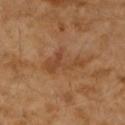<case>
<biopsy_status>not biopsied; imaged during a skin examination</biopsy_status>
<site>right forearm</site>
<lesion_size>
  <long_diameter_mm_approx>5.5</long_diameter_mm_approx>
</lesion_size>
<image>
  <source>total-body photography crop</source>
  <field_of_view_mm>15</field_of_view_mm>
</image>
<lighting>cross-polarized</lighting>
<patient>
  <sex>female</sex>
  <age_approx>70</age_approx>
</patient>
</case>